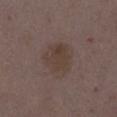The lesion was tiled from a total-body skin photograph and was not biopsied. A region of skin cropped from a whole-body photographic capture, roughly 15 mm wide. Located on the right thigh. The patient is a female approximately 35 years of age.